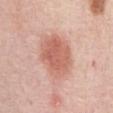Imaged during a routine full-body skin examination; the lesion was not biopsied and no histopathology is available. A 15 mm close-up extracted from a 3D total-body photography capture. A female patient, approximately 65 years of age. From the chest.